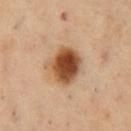Notes:
– notes: catalogued during a skin exam; not biopsied
– image source: 15 mm crop, total-body photography
– TBP lesion metrics: lesion-presence confidence of about 100/100
– diameter: about 4 mm
– illumination: cross-polarized illumination
– subject: male, aged 48–52
– site: the chest